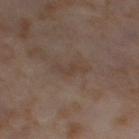No biopsy was performed on this lesion — it was imaged during a full skin examination and was not determined to be concerning. A female subject, in their mid-50s. A region of skin cropped from a whole-body photographic capture, roughly 15 mm wide. On the right thigh.